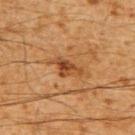Clinical impression: This lesion was catalogued during total-body skin photography and was not selected for biopsy. Acquisition and patient details: A male patient, aged around 60. From the upper back. A 15 mm close-up tile from a total-body photography series done for melanoma screening.